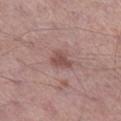Context: The lesion's longest dimension is about 3 mm. A male patient about 55 years old. Captured under white-light illumination. A region of skin cropped from a whole-body photographic capture, roughly 15 mm wide. On the leg. Automated image analysis of the tile measured an area of roughly 5 mm² and an eccentricity of roughly 0.65. The software also gave border irregularity of about 3 on a 0–10 scale and a peripheral color-asymmetry measure near 0.5.